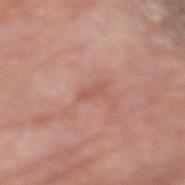Q: Was a biopsy performed?
A: imaged on a skin check; not biopsied
Q: How was this image acquired?
A: ~15 mm tile from a whole-body skin photo
Q: Who is the patient?
A: male, in their 80s
Q: Automated lesion metrics?
A: an area of roughly 2 mm², an eccentricity of roughly 0.9, and a shape-asymmetry score of about 0.4 (0 = symmetric); a mean CIELAB color near L≈55 a*≈25 b*≈29 and about 7 CIELAB-L* units darker than the surrounding skin; border irregularity of about 4 on a 0–10 scale, a color-variation rating of about 0/10, and a peripheral color-asymmetry measure near 0
Q: Lesion size?
A: ~2.5 mm (longest diameter)
Q: What lighting was used for the tile?
A: white-light
Q: What is the anatomic site?
A: the left forearm